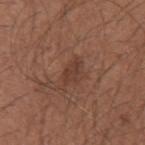notes: no biopsy performed (imaged during a skin exam)
illumination: white-light
body site: the left upper arm
image-analysis metrics: a lesion area of about 3.5 mm² and a shape-asymmetry score of about 0.3 (0 = symmetric); a within-lesion color-variation index near 1/10 and radial color variation of about 0.5
imaging modality: ~15 mm tile from a whole-body skin photo
diameter: ≈3 mm
patient: male, aged 33–37